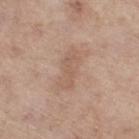Clinical impression: Recorded during total-body skin imaging; not selected for excision or biopsy. Image and clinical context: A female subject approximately 55 years of age. Longest diameter approximately 4.5 mm. A roughly 15 mm field-of-view crop from a total-body skin photograph. Located on the right thigh. Captured under white-light illumination.